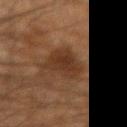| feature | finding |
|---|---|
| diameter | ~5 mm (longest diameter) |
| tile lighting | cross-polarized |
| subject | male, in their 50s |
| automated metrics | an area of roughly 10 mm², an eccentricity of roughly 0.7, and two-axis asymmetry of about 0.4; border irregularity of about 4.5 on a 0–10 scale, a color-variation rating of about 2.5/10, and a peripheral color-asymmetry measure near 1; an automated nevus-likeness rating near 10 out of 100 |
| site | the left forearm |
| imaging modality | 15 mm crop, total-body photography |